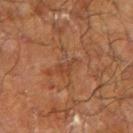No biopsy was performed on this lesion — it was imaged during a full skin examination and was not determined to be concerning.